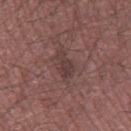The lesion was tiled from a total-body skin photograph and was not biopsied. On the leg. Cropped from a whole-body photographic skin survey; the tile spans about 15 mm. This is a white-light tile. The patient is a male roughly 50 years of age. Longest diameter approximately 3.5 mm.